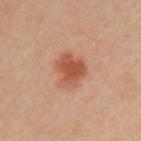Captured during whole-body skin photography for melanoma surveillance; the lesion was not biopsied. From the left upper arm. A lesion tile, about 15 mm wide, cut from a 3D total-body photograph. Automated tile analysis of the lesion measured a color-variation rating of about 3.5/10. A female patient roughly 40 years of age.